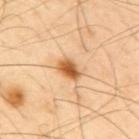The lesion was photographed on a routine skin check and not biopsied; there is no pathology result. The lesion-visualizer software estimated a mean CIELAB color near L≈61 a*≈24 b*≈43, a lesion–skin lightness drop of about 16, and a normalized lesion–skin contrast near 10.5. The software also gave a border-irregularity index near 2/10, a color-variation rating of about 4.5/10, and radial color variation of about 1. It also reported a classifier nevus-likeness of about 100/100 and lesion-presence confidence of about 100/100. A 15 mm crop from a total-body photograph taken for skin-cancer surveillance. A male subject aged approximately 40. The tile uses cross-polarized illumination. On the upper back. Approximately 3 mm at its widest.